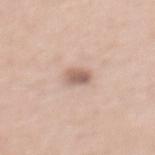Part of a total-body skin-imaging series; this lesion was reviewed on a skin check and was not flagged for biopsy. Longest diameter approximately 2.5 mm. A region of skin cropped from a whole-body photographic capture, roughly 15 mm wide. A female subject aged 58–62. From the upper back.